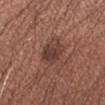Clinical impression: Part of a total-body skin-imaging series; this lesion was reviewed on a skin check and was not flagged for biopsy. Image and clinical context: Cropped from a whole-body photographic skin survey; the tile spans about 15 mm. Approximately 3.5 mm at its widest. A male patient approximately 35 years of age. From the left forearm.